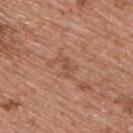Q: Was this lesion biopsied?
A: imaged on a skin check; not biopsied
Q: Where on the body is the lesion?
A: the upper back
Q: Who is the patient?
A: male, aged 53–57
Q: What is the imaging modality?
A: ~15 mm tile from a whole-body skin photo
Q: Lesion size?
A: about 2.5 mm